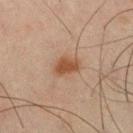{
  "biopsy_status": "not biopsied; imaged during a skin examination",
  "image": {
    "source": "total-body photography crop",
    "field_of_view_mm": 15
  },
  "site": "right thigh",
  "lesion_size": {
    "long_diameter_mm_approx": 3.0
  },
  "patient": {
    "sex": "male",
    "age_approx": 45
  },
  "lighting": "cross-polarized"
}